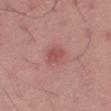| feature | finding |
|---|---|
| notes | imaged on a skin check; not biopsied |
| patient | male, about 45 years old |
| TBP lesion metrics | a lesion color around L≈51 a*≈28 b*≈23 in CIELAB and a normalized lesion–skin contrast near 6; radial color variation of about 1 |
| size | ~3 mm (longest diameter) |
| illumination | white-light |
| image | ~15 mm tile from a whole-body skin photo |
| location | the left thigh |